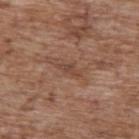Impression: No biopsy was performed on this lesion — it was imaged during a full skin examination and was not determined to be concerning.